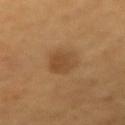<lesion>
<biopsy_status>not biopsied; imaged during a skin examination</biopsy_status>
<image>
  <source>total-body photography crop</source>
  <field_of_view_mm>15</field_of_view_mm>
</image>
<patient>
  <sex>male</sex>
  <age_approx>65</age_approx>
</patient>
<lighting>cross-polarized</lighting>
<site>arm</site>
</lesion>